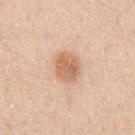<record>
  <image>
    <source>total-body photography crop</source>
    <field_of_view_mm>15</field_of_view_mm>
  </image>
  <patient>
    <sex>male</sex>
    <age_approx>30</age_approx>
  </patient>
  <site>left upper arm</site>
  <lighting>white-light</lighting>
</record>